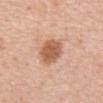Clinical impression: Imaged during a routine full-body skin examination; the lesion was not biopsied and no histopathology is available. Acquisition and patient details: The tile uses white-light illumination. Located on the front of the torso. The subject is a female aged 43 to 47. This image is a 15 mm lesion crop taken from a total-body photograph. Longest diameter approximately 3.5 mm.